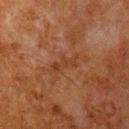biopsy status: imaged on a skin check; not biopsied | lesion size: about 3.5 mm | anatomic site: the chest | subject: male, aged approximately 80 | imaging modality: total-body-photography crop, ~15 mm field of view | automated lesion analysis: a symmetry-axis asymmetry near 0.4; border irregularity of about 5.5 on a 0–10 scale, a color-variation rating of about 0/10, and radial color variation of about 0; an automated nevus-likeness rating near 0 out of 100 and lesion-presence confidence of about 100/100 | lighting: cross-polarized illumination.